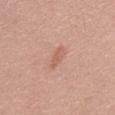<tbp_lesion>
<biopsy_status>not biopsied; imaged during a skin examination</biopsy_status>
<automated_metrics>
  <area_mm2_approx>2.5</area_mm2_approx>
  <eccentricity>0.95</eccentricity>
  <shape_asymmetry>0.2</shape_asymmetry>
</automated_metrics>
<image>
  <source>total-body photography crop</source>
  <field_of_view_mm>15</field_of_view_mm>
</image>
<lesion_size>
  <long_diameter_mm_approx>3.0</long_diameter_mm_approx>
</lesion_size>
<site>front of the torso</site>
<lighting>white-light</lighting>
<patient>
  <sex>male</sex>
  <age_approx>35</age_approx>
</patient>
</tbp_lesion>